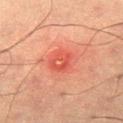Notes:
* notes: imaged on a skin check; not biopsied
* anatomic site: the right thigh
* image: ~15 mm crop, total-body skin-cancer survey
* tile lighting: cross-polarized illumination
* subject: male, in their mid-50s
* image-analysis metrics: a lesion area of about 6 mm², a shape eccentricity near 0.6, and a shape-asymmetry score of about 0.2 (0 = symmetric); a lesion–skin lightness drop of about 8 and a normalized border contrast of about 5.5; internal color variation of about 6 on a 0–10 scale and radial color variation of about 2.5; a nevus-likeness score of about 0/100 and a lesion-detection confidence of about 100/100